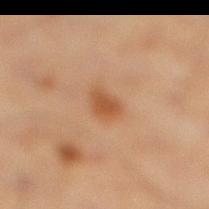{"biopsy_status": "not biopsied; imaged during a skin examination", "automated_metrics": {"area_mm2_approx": 4.5, "shape_asymmetry": 0.3, "lesion_detection_confidence_0_100": 100}, "site": "left lower leg", "patient": {"sex": "male", "age_approx": 60}, "image": {"source": "total-body photography crop", "field_of_view_mm": 15}, "lesion_size": {"long_diameter_mm_approx": 3.0}, "lighting": "cross-polarized"}